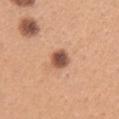{
  "lighting": "white-light",
  "image": {
    "source": "total-body photography crop",
    "field_of_view_mm": 15
  },
  "site": "right upper arm",
  "patient": {
    "sex": "female",
    "age_approx": 30
  }
}